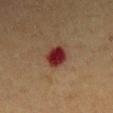Q: Patient demographics?
A: male, aged 58–62
Q: What is the anatomic site?
A: the right upper arm
Q: How was this image acquired?
A: ~15 mm tile from a whole-body skin photo